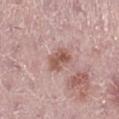Clinical impression:
The lesion was photographed on a routine skin check and not biopsied; there is no pathology result.
Acquisition and patient details:
About 3.5 mm across. Automated tile analysis of the lesion measured a within-lesion color-variation index near 4.5/10. The lesion is located on the right lower leg. A 15 mm close-up tile from a total-body photography series done for melanoma screening. This is a white-light tile. A female subject, roughly 40 years of age.